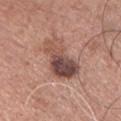biopsy status = catalogued during a skin exam; not biopsied | acquisition = ~15 mm tile from a whole-body skin photo | site = the front of the torso | lesion diameter = about 6 mm | lighting = white-light illumination | subject = male, in their mid-40s.